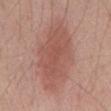Clinical impression: This lesion was catalogued during total-body skin photography and was not selected for biopsy. Context: Cropped from a total-body skin-imaging series; the visible field is about 15 mm. The subject is a male roughly 70 years of age. Captured under white-light illumination. An algorithmic analysis of the crop reported a lesion area of about 30 mm² and two-axis asymmetry of about 0.2. The software also gave a border-irregularity rating of about 3/10, a color-variation rating of about 3/10, and peripheral color asymmetry of about 1. The analysis additionally found a nevus-likeness score of about 95/100 and a detector confidence of about 100 out of 100 that the crop contains a lesion. From the mid back. Measured at roughly 9.5 mm in maximum diameter.